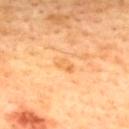<case>
<biopsy_status>not biopsied; imaged during a skin examination</biopsy_status>
<patient>
  <sex>male</sex>
  <age_approx>45</age_approx>
</patient>
<image>
  <source>total-body photography crop</source>
  <field_of_view_mm>15</field_of_view_mm>
</image>
<site>upper back</site>
<lesion_size>
  <long_diameter_mm_approx>2.5</long_diameter_mm_approx>
</lesion_size>
<lighting>cross-polarized</lighting>
</case>